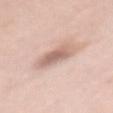workup: catalogued during a skin exam; not biopsied.